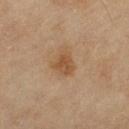Notes:
- biopsy status · catalogued during a skin exam; not biopsied
- patient · female, in their 60s
- tile lighting · cross-polarized illumination
- automated lesion analysis · an area of roughly 5.5 mm² and two-axis asymmetry of about 0.25; border irregularity of about 2 on a 0–10 scale, a within-lesion color-variation index near 2/10, and peripheral color asymmetry of about 0.5
- image source · 15 mm crop, total-body photography
- body site · the left thigh